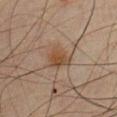Clinical impression: The lesion was tiled from a total-body skin photograph and was not biopsied. Image and clinical context: On the chest. Cropped from a whole-body photographic skin survey; the tile spans about 15 mm. The patient is a male roughly 35 years of age.